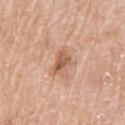Q: Was this lesion biopsied?
A: total-body-photography surveillance lesion; no biopsy
Q: How was this image acquired?
A: 15 mm crop, total-body photography
Q: Who is the patient?
A: male, aged 68 to 72
Q: Lesion location?
A: the left upper arm